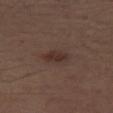Assessment: Recorded during total-body skin imaging; not selected for excision or biopsy. Clinical summary: The recorded lesion diameter is about 3 mm. The lesion-visualizer software estimated a detector confidence of about 100 out of 100 that the crop contains a lesion. The patient is a male about 70 years old. Imaged with white-light lighting. The lesion is located on the right thigh. A roughly 15 mm field-of-view crop from a total-body skin photograph.